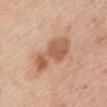| feature | finding |
|---|---|
| notes | imaged on a skin check; not biopsied |
| illumination | white-light illumination |
| acquisition | 15 mm crop, total-body photography |
| TBP lesion metrics | roughly 11 lightness units darker than nearby skin and a normalized lesion–skin contrast near 7.5; a detector confidence of about 100 out of 100 that the crop contains a lesion |
| location | the upper back |
| subject | female, in their 50s |